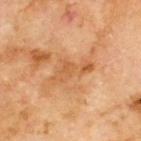Q: Is there a histopathology result?
A: catalogued during a skin exam; not biopsied
Q: Lesion location?
A: the upper back
Q: Illumination type?
A: cross-polarized illumination
Q: What kind of image is this?
A: ~15 mm tile from a whole-body skin photo
Q: What are the patient's age and sex?
A: male, in their 70s